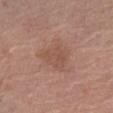Captured during whole-body skin photography for melanoma surveillance; the lesion was not biopsied. Located on the left thigh. Captured under white-light illumination. The patient is a female in their mid-80s or older. A region of skin cropped from a whole-body photographic capture, roughly 15 mm wide.